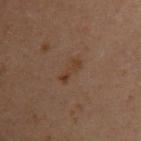• biopsy status: no biopsy performed (imaged during a skin exam)
• body site: the left arm
• automated metrics: an area of roughly 4.5 mm², a shape eccentricity near 0.9, and a symmetry-axis asymmetry near 0.25; a lesion color around L≈30 a*≈14 b*≈23 in CIELAB and a lesion–skin lightness drop of about 4
• patient: female, in their 30s
• image: 15 mm crop, total-body photography
• lesion size: ≈3.5 mm